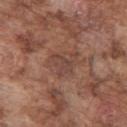follow-up: no biopsy performed (imaged during a skin exam)
body site: the arm
automated metrics: a mean CIELAB color near L≈43 a*≈20 b*≈25, a lesion–skin lightness drop of about 6, and a lesion-to-skin contrast of about 5 (normalized; higher = more distinct)
lesion size: about 3.5 mm
tile lighting: white-light illumination
subject: male, aged around 75
image: total-body-photography crop, ~15 mm field of view